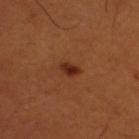Case summary:
• notes — imaged on a skin check; not biopsied
• site — the right thigh
• illumination — cross-polarized
• TBP lesion metrics — a mean CIELAB color near L≈29 a*≈25 b*≈31, about 10 CIELAB-L* units darker than the surrounding skin, and a lesion-to-skin contrast of about 9.5 (normalized; higher = more distinct); a nevus-likeness score of about 100/100 and a detector confidence of about 100 out of 100 that the crop contains a lesion
• lesion size — ~2.5 mm (longest diameter)
• patient — male, approximately 50 years of age
• imaging modality — 15 mm crop, total-body photography